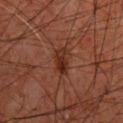workup = total-body-photography surveillance lesion; no biopsy
lighting = cross-polarized
patient = male, aged 58–62
diameter = ≈3.5 mm
anatomic site = the upper back
imaging modality = ~15 mm tile from a whole-body skin photo